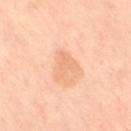{
  "biopsy_status": "not biopsied; imaged during a skin examination",
  "patient": {
    "sex": "female",
    "age_approx": 50
  },
  "image": {
    "source": "total-body photography crop",
    "field_of_view_mm": 15
  },
  "lighting": "cross-polarized",
  "automated_metrics": {
    "color_variation_0_10": 1.0,
    "peripheral_color_asymmetry": 0.5,
    "nevus_likeness_0_100": 0,
    "lesion_detection_confidence_0_100": 100
  },
  "site": "right thigh"
}